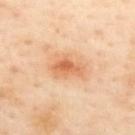biopsy status: catalogued during a skin exam; not biopsied
location: the upper back
lighting: cross-polarized illumination
patient: female, aged approximately 40
lesion size: ≈4 mm
automated lesion analysis: a lesion–skin lightness drop of about 11 and a lesion-to-skin contrast of about 7 (normalized; higher = more distinct); border irregularity of about 4 on a 0–10 scale
imaging modality: 15 mm crop, total-body photography